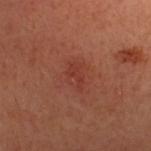Clinical impression:
Captured during whole-body skin photography for melanoma surveillance; the lesion was not biopsied.
Acquisition and patient details:
A close-up tile cropped from a whole-body skin photograph, about 15 mm across. The tile uses cross-polarized illumination. The subject is a female aged 48 to 52. On the head or neck.